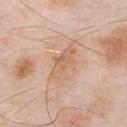Findings:
- notes · no biopsy performed (imaged during a skin exam)
- subject · male, about 55 years old
- tile lighting · white-light
- site · the front of the torso
- image · ~15 mm crop, total-body skin-cancer survey
- size · about 4.5 mm